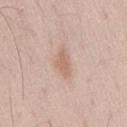workup: total-body-photography surveillance lesion; no biopsy
site: the lower back
automated lesion analysis: an area of roughly 5 mm², an outline eccentricity of about 0.85 (0 = round, 1 = elongated), and a symmetry-axis asymmetry near 0.25; a classifier nevus-likeness of about 25/100 and a detector confidence of about 100 out of 100 that the crop contains a lesion
diameter: ~3.5 mm (longest diameter)
image: ~15 mm crop, total-body skin-cancer survey
tile lighting: white-light
patient: male, about 50 years old